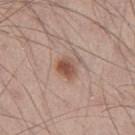| feature | finding |
|---|---|
| follow-up | catalogued during a skin exam; not biopsied |
| location | the left thigh |
| TBP lesion metrics | a lesion area of about 5 mm² and a shape eccentricity near 0.6; a within-lesion color-variation index near 4/10 and radial color variation of about 1; a classifier nevus-likeness of about 90/100 and a detector confidence of about 100 out of 100 that the crop contains a lesion |
| subject | male, aged around 30 |
| imaging modality | 15 mm crop, total-body photography |
| lighting | white-light illumination |
| lesion diameter | ≈2.5 mm |